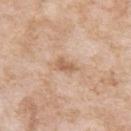This image is a 15 mm lesion crop taken from a total-body photograph. About 3 mm across. The patient is a female aged 73–77. Located on the left upper arm. This is a white-light tile.